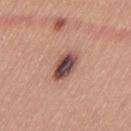Findings:
– biopsy status: total-body-photography surveillance lesion; no biopsy